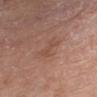Findings:
- biopsy status · total-body-photography surveillance lesion; no biopsy
- image · ~15 mm tile from a whole-body skin photo
- location · the upper back
- subject · female, in their mid-70s